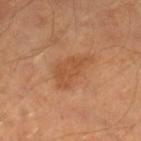workup — total-body-photography surveillance lesion; no biopsy
diameter — ~5 mm (longest diameter)
acquisition — total-body-photography crop, ~15 mm field of view
site — the leg
subject — male, in their 40s
lighting — cross-polarized illumination